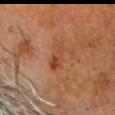notes: total-body-photography surveillance lesion; no biopsy
anatomic site: the head or neck
subject: male, roughly 60 years of age
imaging modality: ~15 mm crop, total-body skin-cancer survey